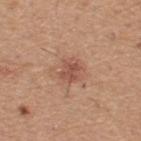notes — no biopsy performed (imaged during a skin exam); subject — male, in their 60s; image — 15 mm crop, total-body photography; lighting — white-light; lesion size — ≈2.5 mm; anatomic site — the upper back.